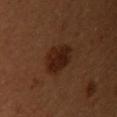biopsy status: catalogued during a skin exam; not biopsied | size: ≈4.5 mm | image: total-body-photography crop, ~15 mm field of view | subject: female, about 50 years old | site: the chest.